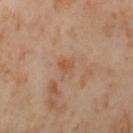Clinical impression: Part of a total-body skin-imaging series; this lesion was reviewed on a skin check and was not flagged for biopsy. Acquisition and patient details: The lesion-visualizer software estimated an area of roughly 3.5 mm², a shape eccentricity near 0.6, and a shape-asymmetry score of about 0.3 (0 = symmetric). The software also gave a border-irregularity index near 2.5/10, internal color variation of about 2.5 on a 0–10 scale, and a peripheral color-asymmetry measure near 1. The patient is a female aged around 55. This image is a 15 mm lesion crop taken from a total-body photograph. The lesion is on the left thigh. Captured under cross-polarized illumination. Longest diameter approximately 2.5 mm.